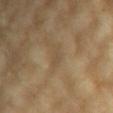Part of a total-body skin-imaging series; this lesion was reviewed on a skin check and was not flagged for biopsy. This image is a 15 mm lesion crop taken from a total-body photograph. An algorithmic analysis of the crop reported a shape eccentricity near 0.9 and a shape-asymmetry score of about 0.3 (0 = symmetric). It also reported a lesion color around L≈48 a*≈13 b*≈31 in CIELAB, roughly 6 lightness units darker than nearby skin, and a lesion-to-skin contrast of about 4.5 (normalized; higher = more distinct). The analysis additionally found a border-irregularity rating of about 3.5/10. And it measured a classifier nevus-likeness of about 0/100 and lesion-presence confidence of about 65/100. Longest diameter approximately 3 mm. On the arm. A female subject, in their mid- to late 70s.